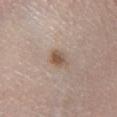Recorded during total-body skin imaging; not selected for excision or biopsy. About 2.5 mm across. A 15 mm close-up extracted from a 3D total-body photography capture. Located on the right lower leg. An algorithmic analysis of the crop reported a footprint of about 4 mm², an outline eccentricity of about 0.65 (0 = round, 1 = elongated), and two-axis asymmetry of about 0.2. The analysis additionally found an average lesion color of about L≈53 a*≈16 b*≈27 (CIELAB). And it measured an automated nevus-likeness rating near 95 out of 100. The tile uses white-light illumination. A female subject roughly 65 years of age.